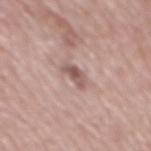Case summary:
* follow-up · no biopsy performed (imaged during a skin exam)
* imaging modality · ~15 mm tile from a whole-body skin photo
* tile lighting · white-light illumination
* diameter · about 3 mm
* location · the back
* subject · male, approximately 70 years of age